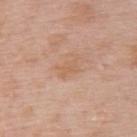biopsy_status: not biopsied; imaged during a skin examination
site: upper back
lighting: white-light
patient:
  sex: female
  age_approx: 50
automated_metrics:
  cielab_L: 61
  cielab_a: 20
  cielab_b: 33
  vs_skin_darker_L: 6.0
lesion_size:
  long_diameter_mm_approx: 3.0
image:
  source: total-body photography crop
  field_of_view_mm: 15